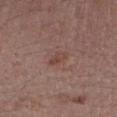  biopsy_status: not biopsied; imaged during a skin examination
  patient:
    sex: female
    age_approx: 55
  site: right forearm
  lighting: white-light
  automated_metrics:
    cielab_L: 44
    cielab_a: 20
    cielab_b: 23
    vs_skin_darker_L: 7.0
    vs_skin_contrast_norm: 5.5
  image:
    source: total-body photography crop
    field_of_view_mm: 15